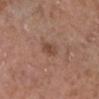Assessment:
The lesion was photographed on a routine skin check and not biopsied; there is no pathology result.
Clinical summary:
Captured under white-light illumination. From the right lower leg. A region of skin cropped from a whole-body photographic capture, roughly 15 mm wide. A male patient, aged around 75.